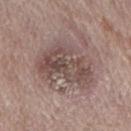workup: no biopsy performed (imaged during a skin exam); location: the mid back; patient: male, aged 68 to 72; imaging modality: ~15 mm tile from a whole-body skin photo.